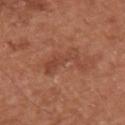workup — no biopsy performed (imaged during a skin exam) | image source — total-body-photography crop, ~15 mm field of view | tile lighting — white-light illumination | subject — male, aged around 65 | lesion size — ≈5.5 mm | body site — the upper back | automated lesion analysis — border irregularity of about 8 on a 0–10 scale, a color-variation rating of about 2/10, and peripheral color asymmetry of about 0.5; a nevus-likeness score of about 0/100 and a lesion-detection confidence of about 100/100.